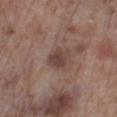Q: What did automated image analysis measure?
A: an outline eccentricity of about 0.35 (0 = round, 1 = elongated) and a shape-asymmetry score of about 0.2 (0 = symmetric); a color-variation rating of about 2/10 and radial color variation of about 0.5; a classifier nevus-likeness of about 30/100 and lesion-presence confidence of about 100/100
Q: What kind of image is this?
A: ~15 mm crop, total-body skin-cancer survey
Q: Who is the patient?
A: male, in their 70s
Q: Where on the body is the lesion?
A: the right lower leg